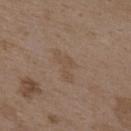Q: Was a biopsy performed?
A: no biopsy performed (imaged during a skin exam)
Q: How was the tile lit?
A: white-light
Q: How was this image acquired?
A: ~15 mm tile from a whole-body skin photo
Q: What are the patient's age and sex?
A: female, aged approximately 35
Q: Lesion location?
A: the upper back